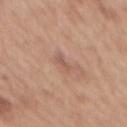This image is a 15 mm lesion crop taken from a total-body photograph.
On the mid back.
A female patient aged approximately 40.
Imaged with white-light lighting.
The lesion-visualizer software estimated an outline eccentricity of about 0.95 (0 = round, 1 = elongated) and a symmetry-axis asymmetry near 0.4. The analysis additionally found a normalized border contrast of about 5.5. And it measured a within-lesion color-variation index near 0/10 and radial color variation of about 0.